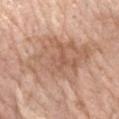biopsy_status: not biopsied; imaged during a skin examination
lighting: white-light
image:
  source: total-body photography crop
  field_of_view_mm: 15
lesion_size:
  long_diameter_mm_approx: 10.5
site: arm
patient:
  sex: female
  age_approx: 65